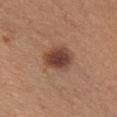<case>
  <biopsy_status>not biopsied; imaged during a skin examination</biopsy_status>
  <patient>
    <sex>female</sex>
    <age_approx>40</age_approx>
  </patient>
  <image>
    <source>total-body photography crop</source>
    <field_of_view_mm>15</field_of_view_mm>
  </image>
  <site>chest</site>
  <lesion_size>
    <long_diameter_mm_approx>4.0</long_diameter_mm_approx>
  </lesion_size>
  <lighting>white-light</lighting>
</case>